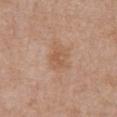Clinical impression: No biopsy was performed on this lesion — it was imaged during a full skin examination and was not determined to be concerning.